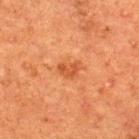{"biopsy_status": "not biopsied; imaged during a skin examination", "site": "upper back", "patient": {"sex": "male", "age_approx": 50}, "automated_metrics": {"cielab_L": 43, "cielab_a": 27, "cielab_b": 36, "vs_skin_darker_L": 8.0, "vs_skin_contrast_norm": 6.5, "border_irregularity_0_10": 3.5, "color_variation_0_10": 2.0, "peripheral_color_asymmetry": 0.5}, "image": {"source": "total-body photography crop", "field_of_view_mm": 15}, "lighting": "cross-polarized"}